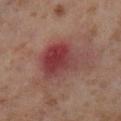Recorded during total-body skin imaging; not selected for excision or biopsy.
A lesion tile, about 15 mm wide, cut from a 3D total-body photograph.
Captured under cross-polarized illumination.
A female subject, in their mid-50s.
Approximately 6 mm at its widest.
The lesion is located on the left lower leg.
Automated image analysis of the tile measured a shape eccentricity near 0.5 and a shape-asymmetry score of about 0.3 (0 = symmetric). And it measured a lesion color around L≈42 a*≈27 b*≈22 in CIELAB, a lesion–skin lightness drop of about 10, and a normalized border contrast of about 8.5. The analysis additionally found a nevus-likeness score of about 5/100.